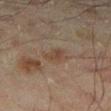Part of a total-body skin-imaging series; this lesion was reviewed on a skin check and was not flagged for biopsy. An algorithmic analysis of the crop reported a lesion area of about 3.5 mm², an eccentricity of roughly 0.8, and a shape-asymmetry score of about 0.3 (0 = symmetric). And it measured a mean CIELAB color near L≈36 a*≈14 b*≈24, a lesion–skin lightness drop of about 6, and a lesion-to-skin contrast of about 6.5 (normalized; higher = more distinct). It also reported a classifier nevus-likeness of about 0/100 and a detector confidence of about 100 out of 100 that the crop contains a lesion. Approximately 2.5 mm at its widest. A 15 mm close-up extracted from a 3D total-body photography capture. Located on the right lower leg. A male patient, in their mid-40s.